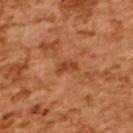subject: female, in their mid-50s
automated lesion analysis: a footprint of about 3 mm², an outline eccentricity of about 0.85 (0 = round, 1 = elongated), and a symmetry-axis asymmetry near 0.4; an average lesion color of about L≈46 a*≈29 b*≈40 (CIELAB), a lesion–skin lightness drop of about 9, and a lesion-to-skin contrast of about 7 (normalized; higher = more distinct); a classifier nevus-likeness of about 0/100 and a detector confidence of about 100 out of 100 that the crop contains a lesion
image: 15 mm crop, total-body photography
body site: the upper back
diameter: about 2.5 mm